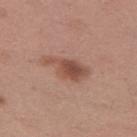A female patient about 30 years old.
A 15 mm close-up extracted from a 3D total-body photography capture.
The lesion is located on the left thigh.
The recorded lesion diameter is about 5.5 mm.